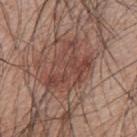workup: no biopsy performed (imaged during a skin exam) | location: the upper back | lesion size: ≈6.5 mm | TBP lesion metrics: a lesion color around L≈44 a*≈21 b*≈24 in CIELAB, a lesion–skin lightness drop of about 8, and a lesion-to-skin contrast of about 6.5 (normalized; higher = more distinct) | image source: 15 mm crop, total-body photography | subject: male, aged 48–52.